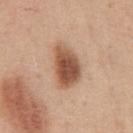On the chest. A male subject, aged around 50. Automated image analysis of the tile measured a border-irregularity rating of about 3/10 and internal color variation of about 5.5 on a 0–10 scale. And it measured an automated nevus-likeness rating near 95 out of 100 and a detector confidence of about 100 out of 100 that the crop contains a lesion. The tile uses white-light illumination. A region of skin cropped from a whole-body photographic capture, roughly 15 mm wide.